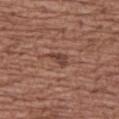The lesion was tiled from a total-body skin photograph and was not biopsied.
Measured at roughly 2.5 mm in maximum diameter.
A roughly 15 mm field-of-view crop from a total-body skin photograph.
The patient is a female aged 73 to 77.
The lesion is on the left thigh.
The tile uses white-light illumination.